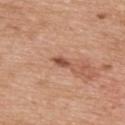Background:
The lesion is located on the upper back. A male subject about 50 years old. Longest diameter approximately 2.5 mm. A 15 mm crop from a total-body photograph taken for skin-cancer surveillance. This is a white-light tile. The lesion-visualizer software estimated an average lesion color of about L≈52 a*≈24 b*≈31 (CIELAB), roughly 12 lightness units darker than nearby skin, and a normalized lesion–skin contrast near 8.5. And it measured internal color variation of about 1 on a 0–10 scale and radial color variation of about 0.5.